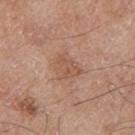Q: Was a biopsy performed?
A: catalogued during a skin exam; not biopsied
Q: What kind of image is this?
A: 15 mm crop, total-body photography
Q: Lesion location?
A: the left thigh
Q: Patient demographics?
A: male, aged around 80
Q: What did automated image analysis measure?
A: a footprint of about 6 mm², an eccentricity of roughly 0.7, and two-axis asymmetry of about 0.35; a within-lesion color-variation index near 2/10 and peripheral color asymmetry of about 0.5
Q: Illumination type?
A: white-light
Q: How large is the lesion?
A: about 3.5 mm